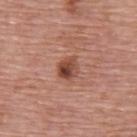Q: Was this lesion biopsied?
A: no biopsy performed (imaged during a skin exam)
Q: What kind of image is this?
A: total-body-photography crop, ~15 mm field of view
Q: Patient demographics?
A: female, aged approximately 65
Q: Lesion location?
A: the upper back